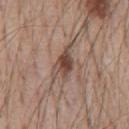Part of a total-body skin-imaging series; this lesion was reviewed on a skin check and was not flagged for biopsy.
The patient is a male about 55 years old.
Cropped from a whole-body photographic skin survey; the tile spans about 15 mm.
Longest diameter approximately 5 mm.
The lesion is on the front of the torso.